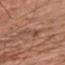Part of a total-body skin-imaging series; this lesion was reviewed on a skin check and was not flagged for biopsy.
A male subject, aged around 65.
This is a white-light tile.
An algorithmic analysis of the crop reported a mean CIELAB color near L≈48 a*≈22 b*≈29, about 6 CIELAB-L* units darker than the surrounding skin, and a normalized border contrast of about 5.
The recorded lesion diameter is about 5 mm.
A roughly 15 mm field-of-view crop from a total-body skin photograph.
The lesion is located on the chest.